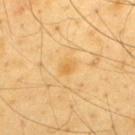Background: The subject is a male aged 63–67. Approximately 2 mm at its widest. A region of skin cropped from a whole-body photographic capture, roughly 15 mm wide. Captured under cross-polarized illumination. On the back.